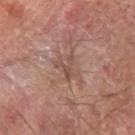Q: Was this lesion biopsied?
A: catalogued during a skin exam; not biopsied
Q: Patient demographics?
A: male, roughly 70 years of age
Q: What kind of image is this?
A: ~15 mm tile from a whole-body skin photo
Q: What is the anatomic site?
A: the left forearm
Q: What did automated image analysis measure?
A: a lesion area of about 4.5 mm² and a shape-asymmetry score of about 0.45 (0 = symmetric); a mean CIELAB color near L≈48 a*≈18 b*≈24, roughly 7 lightness units darker than nearby skin, and a normalized border contrast of about 5.5; an automated nevus-likeness rating near 0 out of 100 and lesion-presence confidence of about 70/100
Q: Illumination type?
A: cross-polarized illumination
Q: How large is the lesion?
A: ~3 mm (longest diameter)